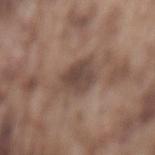diameter — about 4.5 mm | image source — 15 mm crop, total-body photography | anatomic site — the mid back | patient — male, in their mid-70s | TBP lesion metrics — an eccentricity of roughly 0.5 and a symmetry-axis asymmetry near 0.3; a mean CIELAB color near L≈45 a*≈15 b*≈22, a lesion–skin lightness drop of about 10, and a normalized lesion–skin contrast near 8.